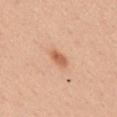Part of a total-body skin-imaging series; this lesion was reviewed on a skin check and was not flagged for biopsy. A roughly 15 mm field-of-view crop from a total-body skin photograph. A female subject, approximately 40 years of age. From the mid back.